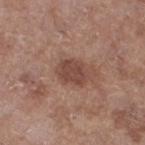| field | value |
|---|---|
| biopsy status | no biopsy performed (imaged during a skin exam) |
| patient | female, approximately 75 years of age |
| automated metrics | a footprint of about 7 mm², a shape eccentricity near 0.65, and two-axis asymmetry of about 0.2; a classifier nevus-likeness of about 40/100 and a detector confidence of about 100 out of 100 that the crop contains a lesion |
| imaging modality | 15 mm crop, total-body photography |
| tile lighting | white-light illumination |
| lesion size | ≈3.5 mm |
| body site | the left lower leg |